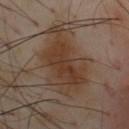notes=imaged on a skin check; not biopsied | lesion size=≈9 mm | automated lesion analysis=an average lesion color of about L≈38 a*≈15 b*≈26 (CIELAB), about 8 CIELAB-L* units darker than the surrounding skin, and a normalized lesion–skin contrast near 8 | anatomic site=the chest | patient=male, in their mid-50s | image source=15 mm crop, total-body photography.